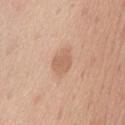Background: A 15 mm close-up extracted from a 3D total-body photography capture. On the chest. Longest diameter approximately 3 mm. A male patient aged 58 to 62.